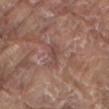Q: Is there a histopathology result?
A: total-body-photography surveillance lesion; no biopsy
Q: How was this image acquired?
A: total-body-photography crop, ~15 mm field of view
Q: What are the patient's age and sex?
A: male, in their 80s
Q: What is the lesion's diameter?
A: ~3.5 mm (longest diameter)
Q: Automated lesion metrics?
A: a shape eccentricity near 0.95 and a shape-asymmetry score of about 0.3 (0 = symmetric); an average lesion color of about L≈46 a*≈20 b*≈22 (CIELAB) and a normalized border contrast of about 5.5; a border-irregularity rating of about 4/10, internal color variation of about 1 on a 0–10 scale, and radial color variation of about 0
Q: Lesion location?
A: the mid back